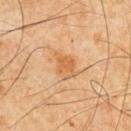follow-up: imaged on a skin check; not biopsied | image: ~15 mm crop, total-body skin-cancer survey | size: ≈3.5 mm | tile lighting: cross-polarized | site: the chest | patient: male, aged 63 to 67.